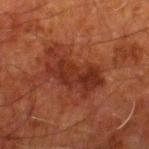Recorded during total-body skin imaging; not selected for excision or biopsy. The lesion is located on the right lower leg. Captured under cross-polarized illumination. A 15 mm crop from a total-body photograph taken for skin-cancer surveillance. The recorded lesion diameter is about 7 mm. A male patient, aged 78 to 82. Automated image analysis of the tile measured roughly 7 lightness units darker than nearby skin. The analysis additionally found a border-irregularity index near 6.5/10, internal color variation of about 3 on a 0–10 scale, and peripheral color asymmetry of about 1.